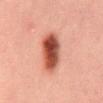biopsy status = no biopsy performed (imaged during a skin exam)
diameter = ~5.5 mm (longest diameter)
automated metrics = an average lesion color of about L≈42 a*≈25 b*≈27 (CIELAB), about 16 CIELAB-L* units darker than the surrounding skin, and a normalized border contrast of about 12; a border-irregularity index near 2/10 and peripheral color asymmetry of about 2; a nevus-likeness score of about 100/100 and lesion-presence confidence of about 100/100
imaging modality = ~15 mm crop, total-body skin-cancer survey
lighting = cross-polarized illumination
patient = male, aged around 30
location = the mid back